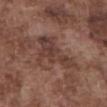Recorded during total-body skin imaging; not selected for excision or biopsy.
Imaged with white-light lighting.
The lesion is located on the abdomen.
The recorded lesion diameter is about 5.5 mm.
A close-up tile cropped from a whole-body skin photograph, about 15 mm across.
The subject is a male about 75 years old.
The total-body-photography lesion software estimated an area of roughly 13 mm², an outline eccentricity of about 0.8 (0 = round, 1 = elongated), and a shape-asymmetry score of about 0.65 (0 = symmetric). The analysis additionally found roughly 8 lightness units darker than nearby skin and a normalized lesion–skin contrast near 7. The analysis additionally found a classifier nevus-likeness of about 0/100 and a detector confidence of about 70 out of 100 that the crop contains a lesion.